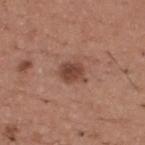Findings:
* biopsy status · no biopsy performed (imaged during a skin exam)
* illumination · white-light
* image source · 15 mm crop, total-body photography
* location · the upper back
* patient · male, aged approximately 65